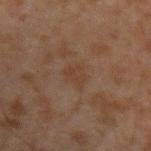biopsy status = catalogued during a skin exam; not biopsied
subject = male, aged 43–47
image = ~15 mm crop, total-body skin-cancer survey
tile lighting = cross-polarized illumination
diameter = about 3 mm
anatomic site = the left forearm